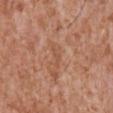The lesion was tiled from a total-body skin photograph and was not biopsied. The tile uses white-light illumination. Cropped from a whole-body photographic skin survey; the tile spans about 15 mm. A male subject, aged approximately 45. The lesion is located on the chest. The total-body-photography lesion software estimated a lesion area of about 2 mm², a shape eccentricity near 0.95, and a symmetry-axis asymmetry near 0.6. The software also gave roughly 6 lightness units darker than nearby skin and a normalized border contrast of about 4.5. The software also gave internal color variation of about 0 on a 0–10 scale and peripheral color asymmetry of about 0. And it measured a nevus-likeness score of about 0/100.